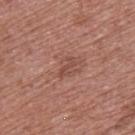Imaged during a routine full-body skin examination; the lesion was not biopsied and no histopathology is available. A male patient, in their mid-50s. A 15 mm close-up extracted from a 3D total-body photography capture. Located on the upper back.